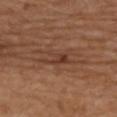Part of a total-body skin-imaging series; this lesion was reviewed on a skin check and was not flagged for biopsy. An algorithmic analysis of the crop reported a footprint of about 6.5 mm² and two-axis asymmetry of about 0.5. The software also gave a mean CIELAB color near L≈39 a*≈22 b*≈29, a lesion–skin lightness drop of about 7, and a normalized border contrast of about 6.5. It also reported a border-irregularity rating of about 8.5/10, a within-lesion color-variation index near 4/10, and a peripheral color-asymmetry measure near 1. The subject is a male aged around 75. This image is a 15 mm lesion crop taken from a total-body photograph. The lesion is on the upper back. The recorded lesion diameter is about 5 mm.